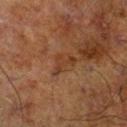The lesion was tiled from a total-body skin photograph and was not biopsied. A region of skin cropped from a whole-body photographic capture, roughly 15 mm wide. The recorded lesion diameter is about 3 mm. The patient is a male aged 68–72. The tile uses cross-polarized illumination. An algorithmic analysis of the crop reported a mean CIELAB color near L≈30 a*≈19 b*≈27, about 6 CIELAB-L* units darker than the surrounding skin, and a lesion-to-skin contrast of about 6 (normalized; higher = more distinct). From the right lower leg.